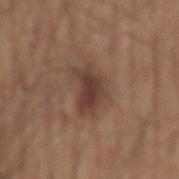Q: Is there a histopathology result?
A: no biopsy performed (imaged during a skin exam)
Q: What kind of image is this?
A: ~15 mm crop, total-body skin-cancer survey
Q: Automated lesion metrics?
A: a footprint of about 10 mm², a shape eccentricity near 0.8, and a shape-asymmetry score of about 0.4 (0 = symmetric); a mean CIELAB color near L≈40 a*≈18 b*≈24, roughly 10 lightness units darker than nearby skin, and a normalized border contrast of about 8.5; an automated nevus-likeness rating near 85 out of 100 and a lesion-detection confidence of about 100/100
Q: Who is the patient?
A: male, approximately 75 years of age
Q: Where on the body is the lesion?
A: the mid back